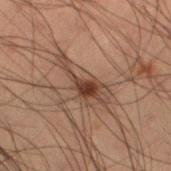notes = imaged on a skin check; not biopsied
lighting = cross-polarized
acquisition = ~15 mm crop, total-body skin-cancer survey
anatomic site = the left lower leg
subject = male, in their mid- to late 50s